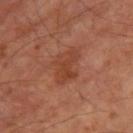{"biopsy_status": "not biopsied; imaged during a skin examination", "automated_metrics": {"area_mm2_approx": 8.0, "eccentricity": 0.8, "shape_asymmetry": 0.35, "cielab_L": 41, "cielab_a": 26, "cielab_b": 31, "vs_skin_darker_L": 7.0, "vs_skin_contrast_norm": 6.0, "color_variation_0_10": 2.5, "peripheral_color_asymmetry": 0.5}, "site": "right thigh", "patient": {"sex": "male", "age_approx": 70}, "lighting": "cross-polarized", "image": {"source": "total-body photography crop", "field_of_view_mm": 15}, "lesion_size": {"long_diameter_mm_approx": 4.5}}